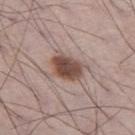Clinical impression: This lesion was catalogued during total-body skin photography and was not selected for biopsy. Background: Located on the left thigh. Automated image analysis of the tile measured a mean CIELAB color near L≈48 a*≈18 b*≈23, a lesion–skin lightness drop of about 15, and a lesion-to-skin contrast of about 10.5 (normalized; higher = more distinct). The analysis additionally found an automated nevus-likeness rating near 95 out of 100 and a detector confidence of about 100 out of 100 that the crop contains a lesion. A male patient in their 70s. Imaged with white-light lighting. A 15 mm crop from a total-body photograph taken for skin-cancer surveillance. Approximately 4 mm at its widest.